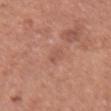Q: Was a biopsy performed?
A: catalogued during a skin exam; not biopsied
Q: What is the imaging modality?
A: ~15 mm crop, total-body skin-cancer survey
Q: What is the anatomic site?
A: the right forearm
Q: What are the patient's age and sex?
A: female, about 50 years old